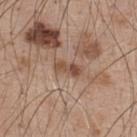Findings:
– follow-up — catalogued during a skin exam; not biopsied
– diameter — about 3.5 mm
– subject — male, about 50 years old
– body site — the upper back
– acquisition — ~15 mm tile from a whole-body skin photo